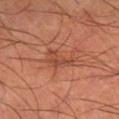Clinical impression:
Captured during whole-body skin photography for melanoma surveillance; the lesion was not biopsied.
Acquisition and patient details:
Measured at roughly 3.5 mm in maximum diameter. The patient is a male aged around 55. Cropped from a whole-body photographic skin survey; the tile spans about 15 mm. The lesion is on the right lower leg.